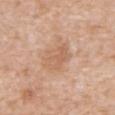| field | value |
|---|---|
| notes | catalogued during a skin exam; not biopsied |
| subject | male, roughly 60 years of age |
| site | the mid back |
| image source | total-body-photography crop, ~15 mm field of view |